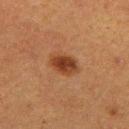The lesion was photographed on a routine skin check and not biopsied; there is no pathology result. A female subject in their 40s. Located on the left thigh. Cropped from a total-body skin-imaging series; the visible field is about 15 mm.